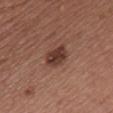No biopsy was performed on this lesion — it was imaged during a full skin examination and was not determined to be concerning. The lesion is on the front of the torso. Imaged with white-light lighting. A female patient roughly 55 years of age. Cropped from a total-body skin-imaging series; the visible field is about 15 mm.